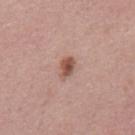{
  "biopsy_status": "not biopsied; imaged during a skin examination",
  "lighting": "white-light",
  "image": {
    "source": "total-body photography crop",
    "field_of_view_mm": 15
  },
  "site": "mid back",
  "patient": {
    "sex": "male",
    "age_approx": 60
  },
  "lesion_size": {
    "long_diameter_mm_approx": 2.5
  }
}